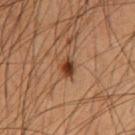This lesion was catalogued during total-body skin photography and was not selected for biopsy. Imaged with cross-polarized lighting. A male patient, in their mid- to late 50s. Cropped from a whole-body photographic skin survey; the tile spans about 15 mm. Located on the chest.